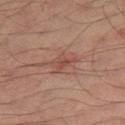Part of a total-body skin-imaging series; this lesion was reviewed on a skin check and was not flagged for biopsy. A 15 mm close-up tile from a total-body photography series done for melanoma screening. Approximately 3 mm at its widest. Located on the right thigh. Imaged with cross-polarized lighting. A male patient, approximately 35 years of age.